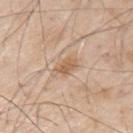Findings:
- follow-up · imaged on a skin check; not biopsied